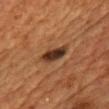<case>
  <biopsy_status>not biopsied; imaged during a skin examination</biopsy_status>
  <patient>
    <sex>male</sex>
    <age_approx>60</age_approx>
  </patient>
  <site>front of the torso</site>
  <lesion_size>
    <long_diameter_mm_approx>3.5</long_diameter_mm_approx>
  </lesion_size>
  <image>
    <source>total-body photography crop</source>
    <field_of_view_mm>15</field_of_view_mm>
  </image>
  <automated_metrics>
    <cielab_L>28</cielab_L>
    <cielab_a>18</cielab_a>
    <cielab_b>25</cielab_b>
    <vs_skin_darker_L>13.0</vs_skin_darker_L>
    <nevus_likeness_0_100>85</nevus_likeness_0_100>
    <lesion_detection_confidence_0_100>100</lesion_detection_confidence_0_100>
  </automated_metrics>
</case>